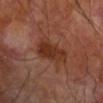The lesion was tiled from a total-body skin photograph and was not biopsied. The lesion is on the right forearm. Cropped from a whole-body photographic skin survey; the tile spans about 15 mm. A male patient about 70 years old.